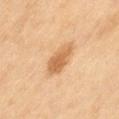Notes:
- follow-up: no biopsy performed (imaged during a skin exam)
- lesion size: ~4 mm (longest diameter)
- lighting: cross-polarized illumination
- acquisition: ~15 mm crop, total-body skin-cancer survey
- subject: female, aged approximately 60
- location: the left thigh